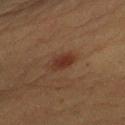Imaged during a routine full-body skin examination; the lesion was not biopsied and no histopathology is available. The tile uses cross-polarized illumination. An algorithmic analysis of the crop reported a color-variation rating of about 2.5/10 and radial color variation of about 1. The lesion's longest dimension is about 3 mm. A 15 mm crop from a total-body photograph taken for skin-cancer surveillance. The lesion is on the left upper arm. A female patient approximately 60 years of age.